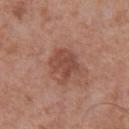Captured during whole-body skin photography for melanoma surveillance; the lesion was not biopsied.
The patient is a male aged 68 to 72.
Imaged with white-light lighting.
The recorded lesion diameter is about 3.5 mm.
Automated image analysis of the tile measured a lesion area of about 10 mm², an outline eccentricity of about 0.4 (0 = round, 1 = elongated), and a shape-asymmetry score of about 0.25 (0 = symmetric). It also reported a border-irregularity index near 3/10. And it measured a classifier nevus-likeness of about 0/100 and a detector confidence of about 100 out of 100 that the crop contains a lesion.
A roughly 15 mm field-of-view crop from a total-body skin photograph.
The lesion is located on the front of the torso.